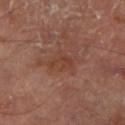biopsy_status: not biopsied; imaged during a skin examination
site: left lower leg
image:
  source: total-body photography crop
  field_of_view_mm: 15
lesion_size:
  long_diameter_mm_approx: 3.5
patient:
  sex: male
  age_approx: 70
automated_metrics:
  border_irregularity_0_10: 6.0
  color_variation_0_10: 1.5
  peripheral_color_asymmetry: 0.5
lighting: cross-polarized